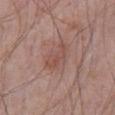Assessment:
Imaged during a routine full-body skin examination; the lesion was not biopsied and no histopathology is available.
Clinical summary:
A 15 mm crop from a total-body photograph taken for skin-cancer surveillance. An algorithmic analysis of the crop reported an area of roughly 7 mm² and an outline eccentricity of about 0.8 (0 = round, 1 = elongated). And it measured a lesion color around L≈50 a*≈20 b*≈24 in CIELAB and roughly 7 lightness units darker than nearby skin. The software also gave border irregularity of about 3 on a 0–10 scale, a within-lesion color-variation index near 3.5/10, and radial color variation of about 1. The software also gave a nevus-likeness score of about 0/100. The lesion is on the abdomen. The recorded lesion diameter is about 4 mm. The tile uses white-light illumination. A male subject aged around 70.